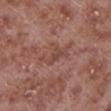– biopsy status: total-body-photography surveillance lesion; no biopsy
– lesion diameter: ≈4 mm
– patient: male, in their mid-50s
– image source: total-body-photography crop, ~15 mm field of view
– anatomic site: the left lower leg